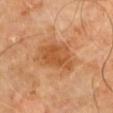Assessment: Imaged during a routine full-body skin examination; the lesion was not biopsied and no histopathology is available. Background: Approximately 4.5 mm at its widest. The patient is a male approximately 60 years of age. A close-up tile cropped from a whole-body skin photograph, about 15 mm across. Located on the upper back. The tile uses cross-polarized illumination.